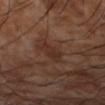biopsy_status: not biopsied; imaged during a skin examination
site: left forearm
image:
  source: total-body photography crop
  field_of_view_mm: 15
lesion_size:
  long_diameter_mm_approx: 3.5
patient:
  sex: male
  age_approx: 70
automated_metrics:
  area_mm2_approx: 5.5
  eccentricity: 0.85
  shape_asymmetry: 0.25
  color_variation_0_10: 2.5
  peripheral_color_asymmetry: 0.5
  nevus_likeness_0_100: 0